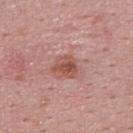  biopsy_status: not biopsied; imaged during a skin examination
  site: upper back
  patient:
    sex: male
    age_approx: 25
  lighting: white-light
  image:
    source: total-body photography crop
    field_of_view_mm: 15
  automated_metrics:
    area_mm2_approx: 5.5
    eccentricity: 0.45
    shape_asymmetry: 0.35